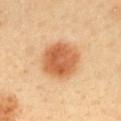follow-up: total-body-photography surveillance lesion; no biopsy
tile lighting: cross-polarized
imaging modality: 15 mm crop, total-body photography
TBP lesion metrics: a lesion area of about 18 mm² and a shape-asymmetry score of about 0.1 (0 = symmetric); a nevus-likeness score of about 100/100 and lesion-presence confidence of about 100/100
location: the upper back
patient: female, about 40 years old
size: ≈5 mm